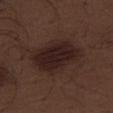No biopsy was performed on this lesion — it was imaged during a full skin examination and was not determined to be concerning. The recorded lesion diameter is about 7 mm. Cropped from a whole-body photographic skin survey; the tile spans about 15 mm. The total-body-photography lesion software estimated a lesion–skin lightness drop of about 8 and a lesion-to-skin contrast of about 10.5 (normalized; higher = more distinct). It also reported a border-irregularity rating of about 2.5/10, internal color variation of about 3.5 on a 0–10 scale, and a peripheral color-asymmetry measure near 1. Located on the right thigh. The patient is a male about 70 years old.